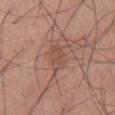acquisition: ~15 mm crop, total-body skin-cancer survey | subject: male, in their mid-50s | site: the front of the torso | lighting: white-light illumination.